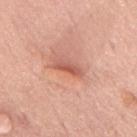notes — total-body-photography surveillance lesion; no biopsy
lesion diameter — about 3.5 mm
subject — male, approximately 75 years of age
anatomic site — the mid back
illumination — white-light illumination
imaging modality — ~15 mm tile from a whole-body skin photo